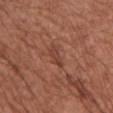workup: no biopsy performed (imaged during a skin exam) | acquisition: total-body-photography crop, ~15 mm field of view | subject: female, aged 73–77 | anatomic site: the chest | size: ~3 mm (longest diameter) | TBP lesion metrics: a footprint of about 3.5 mm²; a lesion–skin lightness drop of about 7 and a lesion-to-skin contrast of about 6 (normalized; higher = more distinct); a border-irregularity index near 3.5/10, internal color variation of about 0.5 on a 0–10 scale, and a peripheral color-asymmetry measure near 0; a classifier nevus-likeness of about 0/100 and lesion-presence confidence of about 90/100 | illumination: white-light illumination.